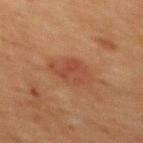patient — male, about 50 years old; imaging modality — ~15 mm tile from a whole-body skin photo; site — the mid back.